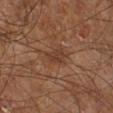Impression: No biopsy was performed on this lesion — it was imaged during a full skin examination and was not determined to be concerning. Clinical summary: The lesion-visualizer software estimated a lesion area of about 4 mm² and an outline eccentricity of about 0.65 (0 = round, 1 = elongated). The software also gave a mean CIELAB color near L≈36 a*≈20 b*≈27 and a lesion-to-skin contrast of about 6 (normalized; higher = more distinct). The software also gave a nevus-likeness score of about 0/100 and lesion-presence confidence of about 100/100. A male subject aged 58 to 62. This is a cross-polarized tile. The recorded lesion diameter is about 2.5 mm. On the right leg. This image is a 15 mm lesion crop taken from a total-body photograph.